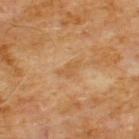The lesion was tiled from a total-body skin photograph and was not biopsied. A male subject, about 60 years old. Longest diameter approximately 3 mm. A region of skin cropped from a whole-body photographic capture, roughly 15 mm wide. The lesion is located on the chest.